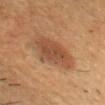| field | value |
|---|---|
| biopsy status | imaged on a skin check; not biopsied |
| automated metrics | an average lesion color of about L≈43 a*≈20 b*≈30 (CIELAB), a lesion–skin lightness drop of about 9, and a lesion-to-skin contrast of about 7.5 (normalized; higher = more distinct); an automated nevus-likeness rating near 100 out of 100 and a detector confidence of about 100 out of 100 that the crop contains a lesion |
| image source | 15 mm crop, total-body photography |
| lesion size | about 6 mm |
| anatomic site | the head or neck |
| tile lighting | cross-polarized illumination |
| patient | male, in their 40s |